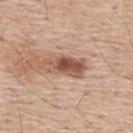{"biopsy_status": "not biopsied; imaged during a skin examination", "patient": {"sex": "male", "age_approx": 55}, "lighting": "white-light", "image": {"source": "total-body photography crop", "field_of_view_mm": 15}, "lesion_size": {"long_diameter_mm_approx": 5.0}, "site": "upper back"}